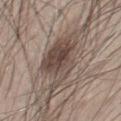<tbp_lesion>
  <biopsy_status>not biopsied; imaged during a skin examination</biopsy_status>
  <patient>
    <sex>male</sex>
    <age_approx>45</age_approx>
  </patient>
  <lighting>white-light</lighting>
  <lesion_size>
    <long_diameter_mm_approx>7.5</long_diameter_mm_approx>
  </lesion_size>
  <image>
    <source>total-body photography crop</source>
    <field_of_view_mm>15</field_of_view_mm>
  </image>
  <site>chest</site>
</tbp_lesion>